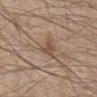{"biopsy_status": "not biopsied; imaged during a skin examination"}